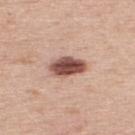An algorithmic analysis of the crop reported roughly 19 lightness units darker than nearby skin and a lesion-to-skin contrast of about 12.5 (normalized; higher = more distinct).
A 15 mm close-up tile from a total-body photography series done for melanoma screening.
About 4.5 mm across.
The subject is a male aged 73–77.
On the upper back.
Captured under white-light illumination.